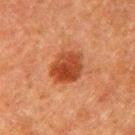No biopsy was performed on this lesion — it was imaged during a full skin examination and was not determined to be concerning. The recorded lesion diameter is about 4.5 mm. A 15 mm close-up tile from a total-body photography series done for melanoma screening. The subject is a male aged 58 to 62. From the right upper arm. An algorithmic analysis of the crop reported a lesion area of about 12 mm², an outline eccentricity of about 0.6 (0 = round, 1 = elongated), and a shape-asymmetry score of about 0.15 (0 = symmetric). And it measured a lesion color around L≈36 a*≈25 b*≈32 in CIELAB. And it measured a border-irregularity rating of about 1.5/10 and radial color variation of about 1.5.